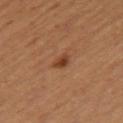The lesion was tiled from a total-body skin photograph and was not biopsied.
A roughly 15 mm field-of-view crop from a total-body skin photograph.
An algorithmic analysis of the crop reported an area of roughly 3 mm², an outline eccentricity of about 0.7 (0 = round, 1 = elongated), and two-axis asymmetry of about 0.3. And it measured an automated nevus-likeness rating near 80 out of 100 and lesion-presence confidence of about 100/100.
A female subject aged approximately 55.
Located on the right thigh.
The tile uses cross-polarized illumination.